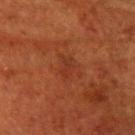notes: imaged on a skin check; not biopsied | patient: female, about 80 years old | location: the head or neck | tile lighting: cross-polarized | diameter: ≈3 mm | image: ~15 mm tile from a whole-body skin photo | automated metrics: an average lesion color of about L≈26 a*≈22 b*≈27 (CIELAB), a lesion–skin lightness drop of about 4, and a lesion-to-skin contrast of about 4.5 (normalized; higher = more distinct); a border-irregularity index near 3.5/10, internal color variation of about 2.5 on a 0–10 scale, and radial color variation of about 1; a nevus-likeness score of about 0/100 and a detector confidence of about 100 out of 100 that the crop contains a lesion.